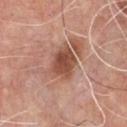The lesion was photographed on a routine skin check and not biopsied; there is no pathology result. This is a white-light tile. Longest diameter approximately 4 mm. The lesion is on the chest. A 15 mm crop from a total-body photograph taken for skin-cancer surveillance. A male subject about 70 years old. The total-body-photography lesion software estimated a lesion area of about 9 mm², a shape eccentricity near 0.65, and a symmetry-axis asymmetry near 0.2.